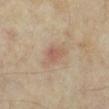workup: no biopsy performed (imaged during a skin exam); lesion diameter: about 3 mm; body site: the left lower leg; subject: female, approximately 35 years of age; illumination: cross-polarized illumination; image: total-body-photography crop, ~15 mm field of view.